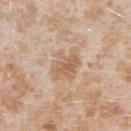lighting: white-light
TBP lesion metrics: a lesion area of about 10 mm², an outline eccentricity of about 0.65 (0 = round, 1 = elongated), and two-axis asymmetry of about 0.25; a mean CIELAB color near L≈62 a*≈16 b*≈32; internal color variation of about 3.5 on a 0–10 scale and radial color variation of about 1.5
lesion size: ~4.5 mm (longest diameter)
image source: total-body-photography crop, ~15 mm field of view
anatomic site: the right forearm
patient: female, in their mid-20s